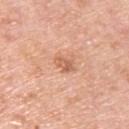Part of a total-body skin-imaging series; this lesion was reviewed on a skin check and was not flagged for biopsy. This is a white-light tile. The subject is a male approximately 80 years of age. A close-up tile cropped from a whole-body skin photograph, about 15 mm across. The lesion is on the upper back. An algorithmic analysis of the crop reported an average lesion color of about L≈62 a*≈25 b*≈34 (CIELAB), roughly 10 lightness units darker than nearby skin, and a normalized border contrast of about 6.5.